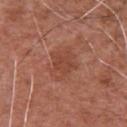{
  "biopsy_status": "not biopsied; imaged during a skin examination",
  "lighting": "white-light",
  "patient": {
    "sex": "male",
    "age_approx": 75
  },
  "automated_metrics": {
    "area_mm2_approx": 11.0,
    "eccentricity": 0.6,
    "nevus_likeness_0_100": 5
  },
  "site": "chest",
  "image": {
    "source": "total-body photography crop",
    "field_of_view_mm": 15
  },
  "lesion_size": {
    "long_diameter_mm_approx": 4.0
  }
}